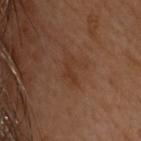Captured during whole-body skin photography for melanoma surveillance; the lesion was not biopsied. A female subject approximately 60 years of age. The lesion's longest dimension is about 2.5 mm. The tile uses cross-polarized illumination. The total-body-photography lesion software estimated an area of roughly 3 mm², an outline eccentricity of about 0.85 (0 = round, 1 = elongated), and a symmetry-axis asymmetry near 0.5. The analysis additionally found roughly 4 lightness units darker than nearby skin and a normalized border contrast of about 5. It also reported a lesion-detection confidence of about 100/100. Located on the upper back. A 15 mm close-up tile from a total-body photography series done for melanoma screening.